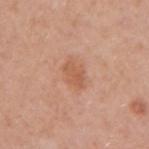Clinical impression: Part of a total-body skin-imaging series; this lesion was reviewed on a skin check and was not flagged for biopsy. Image and clinical context: The lesion is located on the right upper arm. Cropped from a total-body skin-imaging series; the visible field is about 15 mm. This is a white-light tile. Longest diameter approximately 3 mm. The patient is a female aged 48–52. The total-body-photography lesion software estimated a footprint of about 4 mm², an outline eccentricity of about 0.8 (0 = round, 1 = elongated), and a symmetry-axis asymmetry near 0.3. The software also gave internal color variation of about 1.5 on a 0–10 scale and a peripheral color-asymmetry measure near 0.5.